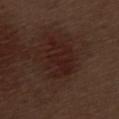<record>
<biopsy_status>not biopsied; imaged during a skin examination</biopsy_status>
<site>left thigh</site>
<patient>
  <sex>male</sex>
  <age_approx>70</age_approx>
</patient>
<image>
  <source>total-body photography crop</source>
  <field_of_view_mm>15</field_of_view_mm>
</image>
<lighting>white-light</lighting>
<automated_metrics>
  <cielab_L>21</cielab_L>
  <cielab_a>19</cielab_a>
  <cielab_b>20</cielab_b>
  <vs_skin_darker_L>5.0</vs_skin_darker_L>
  <vs_skin_contrast_norm>6.5</vs_skin_contrast_norm>
  <border_irregularity_0_10>5.0</border_irregularity_0_10>
  <color_variation_0_10>3.0</color_variation_0_10>
  <peripheral_color_asymmetry>1.0</peripheral_color_asymmetry>
  <nevus_likeness_0_100>5</nevus_likeness_0_100>
  <lesion_detection_confidence_0_100>100</lesion_detection_confidence_0_100>
</automated_metrics>
<lesion_size>
  <long_diameter_mm_approx>5.0</long_diameter_mm_approx>
</lesion_size>
</record>